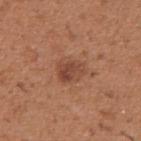Q: Is there a histopathology result?
A: catalogued during a skin exam; not biopsied
Q: What are the patient's age and sex?
A: male, aged approximately 40
Q: Illumination type?
A: white-light
Q: How was this image acquired?
A: ~15 mm tile from a whole-body skin photo
Q: Where on the body is the lesion?
A: the right upper arm